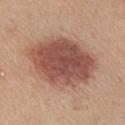body site: the chest; subject: female, approximately 45 years of age; acquisition: 15 mm crop, total-body photography.